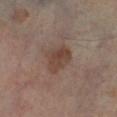{"patient": {"sex": "male", "age_approx": 65}, "lighting": "cross-polarized", "image": {"source": "total-body photography crop", "field_of_view_mm": 15}, "lesion_size": {"long_diameter_mm_approx": 3.5}, "site": "right lower leg"}